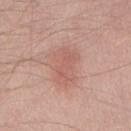Impression:
Part of a total-body skin-imaging series; this lesion was reviewed on a skin check and was not flagged for biopsy.
Image and clinical context:
A male subject, aged approximately 40. The lesion is located on the front of the torso. Captured under white-light illumination. A lesion tile, about 15 mm wide, cut from a 3D total-body photograph. An algorithmic analysis of the crop reported an average lesion color of about L≈59 a*≈23 b*≈25 (CIELAB), about 7 CIELAB-L* units darker than the surrounding skin, and a lesion-to-skin contrast of about 4.5 (normalized; higher = more distinct). The software also gave a border-irregularity index near 3/10, a color-variation rating of about 2.5/10, and peripheral color asymmetry of about 1. The analysis additionally found an automated nevus-likeness rating near 5 out of 100 and a lesion-detection confidence of about 100/100.